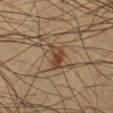The lesion was tiled from a total-body skin photograph and was not biopsied. Located on the left lower leg. Cropped from a whole-body photographic skin survey; the tile spans about 15 mm. A male subject approximately 35 years of age. The lesion-visualizer software estimated border irregularity of about 4.5 on a 0–10 scale and a within-lesion color-variation index near 4/10. Captured under cross-polarized illumination.